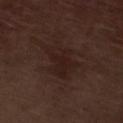The lesion was photographed on a routine skin check and not biopsied; there is no pathology result. The lesion is located on the left lower leg. The tile uses white-light illumination. The subject is a male approximately 70 years of age. Measured at roughly 5 mm in maximum diameter. Cropped from a total-body skin-imaging series; the visible field is about 15 mm. The total-body-photography lesion software estimated a lesion color around L≈19 a*≈15 b*≈18 in CIELAB, a lesion–skin lightness drop of about 5, and a normalized border contrast of about 6. The software also gave a classifier nevus-likeness of about 0/100 and a detector confidence of about 100 out of 100 that the crop contains a lesion.